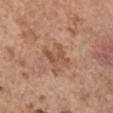| feature | finding |
|---|---|
| notes | no biopsy performed (imaged during a skin exam) |
| lighting | white-light illumination |
| patient | female, aged approximately 65 |
| TBP lesion metrics | border irregularity of about 5.5 on a 0–10 scale and internal color variation of about 2 on a 0–10 scale |
| imaging modality | ~15 mm crop, total-body skin-cancer survey |
| site | the left upper arm |